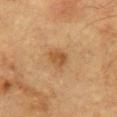The lesion was photographed on a routine skin check and not biopsied; there is no pathology result. A male subject approximately 85 years of age or older. Approximately 2.5 mm at its widest. Cropped from a whole-body photographic skin survey; the tile spans about 15 mm. On the front of the torso.